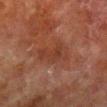Background:
A roughly 15 mm field-of-view crop from a total-body skin photograph. Measured at roughly 4.5 mm in maximum diameter. Automated image analysis of the tile measured a lesion area of about 9.5 mm² and an eccentricity of roughly 0.75. And it measured a normalized lesion–skin contrast near 5.5. It also reported a classifier nevus-likeness of about 0/100 and lesion-presence confidence of about 100/100. On the right lower leg. The subject is a male in their 80s. The tile uses cross-polarized illumination.